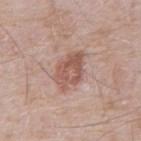No biopsy was performed on this lesion — it was imaged during a full skin examination and was not determined to be concerning. This is a white-light tile. Cropped from a whole-body photographic skin survey; the tile spans about 15 mm. The recorded lesion diameter is about 4.5 mm. On the abdomen. A male subject approximately 80 years of age.